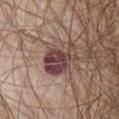The lesion was photographed on a routine skin check and not biopsied; there is no pathology result.
The lesion is on the front of the torso.
The recorded lesion diameter is about 5 mm.
Automated tile analysis of the lesion measured a mean CIELAB color near L≈41 a*≈20 b*≈19, about 14 CIELAB-L* units darker than the surrounding skin, and a normalized lesion–skin contrast near 11.5. It also reported a nevus-likeness score of about 20/100 and a lesion-detection confidence of about 100/100.
A lesion tile, about 15 mm wide, cut from a 3D total-body photograph.
The tile uses white-light illumination.
A male subject aged 63–67.